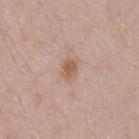Notes:
* biopsy status — total-body-photography surveillance lesion; no biopsy
* image — ~15 mm tile from a whole-body skin photo
* location — the left upper arm
* lesion diameter — about 2.5 mm
* TBP lesion metrics — an area of roughly 4 mm², a shape eccentricity near 0.75, and a shape-asymmetry score of about 0.25 (0 = symmetric); roughly 9 lightness units darker than nearby skin and a normalized border contrast of about 7
* lighting — white-light illumination
* patient — male, in their 40s